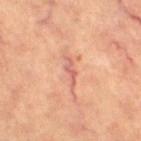workup = total-body-photography surveillance lesion; no biopsy | imaging modality = 15 mm crop, total-body photography | diameter = ~3.5 mm (longest diameter) | patient = female, about 65 years old | anatomic site = the right thigh | illumination = cross-polarized illumination.